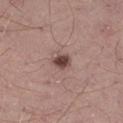notes: imaged on a skin check; not biopsied | location: the right thigh | subject: male, aged 38–42 | diameter: ~2.5 mm (longest diameter) | image source: 15 mm crop, total-body photography | illumination: white-light illumination.